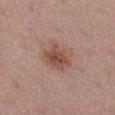A female patient approximately 55 years of age. Located on the left thigh. A lesion tile, about 15 mm wide, cut from a 3D total-body photograph.